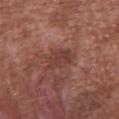Part of a total-body skin-imaging series; this lesion was reviewed on a skin check and was not flagged for biopsy. A region of skin cropped from a whole-body photographic capture, roughly 15 mm wide. The tile uses white-light illumination. A male patient, in their mid- to late 40s. Automated image analysis of the tile measured an average lesion color of about L≈40 a*≈23 b*≈24 (CIELAB), roughly 8 lightness units darker than nearby skin, and a lesion-to-skin contrast of about 6.5 (normalized; higher = more distinct). The analysis additionally found an automated nevus-likeness rating near 0 out of 100 and a detector confidence of about 100 out of 100 that the crop contains a lesion. The lesion is located on the chest.